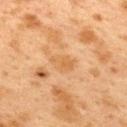| feature | finding |
|---|---|
| notes | no biopsy performed (imaged during a skin exam) |
| image source | total-body-photography crop, ~15 mm field of view |
| tile lighting | cross-polarized illumination |
| subject | female, in their 40s |
| anatomic site | the upper back |